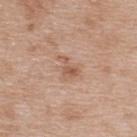{"biopsy_status": "not biopsied; imaged during a skin examination", "site": "back", "lesion_size": {"long_diameter_mm_approx": 3.0}, "patient": {"sex": "female", "age_approx": 40}, "automated_metrics": {"nevus_likeness_0_100": 20, "lesion_detection_confidence_0_100": 100}, "lighting": "white-light", "image": {"source": "total-body photography crop", "field_of_view_mm": 15}}